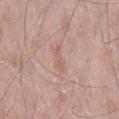image — 15 mm crop, total-body photography; size — ~3.5 mm (longest diameter); tile lighting — white-light; subject — male, aged 48 to 52; site — the right thigh.